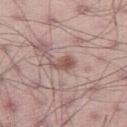Impression: This lesion was catalogued during total-body skin photography and was not selected for biopsy. Image and clinical context: This is a white-light tile. Cropped from a whole-body photographic skin survey; the tile spans about 15 mm. The lesion's longest dimension is about 3 mm. Automated image analysis of the tile measured a lesion area of about 4 mm², an outline eccentricity of about 0.85 (0 = round, 1 = elongated), and two-axis asymmetry of about 0.4. And it measured an average lesion color of about L≈53 a*≈20 b*≈24 (CIELAB), roughly 11 lightness units darker than nearby skin, and a normalized lesion–skin contrast near 8. The software also gave a detector confidence of about 100 out of 100 that the crop contains a lesion. From the right thigh. The patient is a male in their 30s.